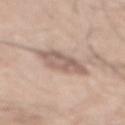| key | value |
|---|---|
| subject | male, in their mid-60s |
| acquisition | ~15 mm crop, total-body skin-cancer survey |
| anatomic site | the back |